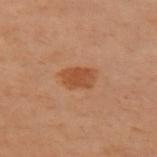follow-up — imaged on a skin check; not biopsied